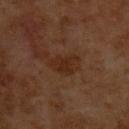Impression: Part of a total-body skin-imaging series; this lesion was reviewed on a skin check and was not flagged for biopsy. Acquisition and patient details: On the upper back. A male patient about 60 years old. Cropped from a total-body skin-imaging series; the visible field is about 15 mm. Automated tile analysis of the lesion measured a lesion area of about 5 mm², an outline eccentricity of about 0.7 (0 = round, 1 = elongated), and a symmetry-axis asymmetry near 0.45. The analysis additionally found a lesion color around L≈24 a*≈18 b*≈26 in CIELAB, about 5 CIELAB-L* units darker than the surrounding skin, and a lesion-to-skin contrast of about 6.5 (normalized; higher = more distinct). The software also gave an automated nevus-likeness rating near 0 out of 100 and a detector confidence of about 100 out of 100 that the crop contains a lesion.